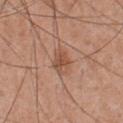Part of a total-body skin-imaging series; this lesion was reviewed on a skin check and was not flagged for biopsy. A 15 mm close-up extracted from a 3D total-body photography capture. From the chest. A male subject aged 53–57. Captured under white-light illumination. Longest diameter approximately 3.5 mm.